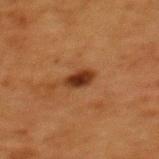Clinical impression:
No biopsy was performed on this lesion — it was imaged during a full skin examination and was not determined to be concerning.
Acquisition and patient details:
A female subject aged 48–52. The lesion's longest dimension is about 3 mm. The lesion is located on the mid back. Automated image analysis of the tile measured a mean CIELAB color near L≈29 a*≈21 b*≈30, a lesion–skin lightness drop of about 12, and a lesion-to-skin contrast of about 11.5 (normalized; higher = more distinct). And it measured a border-irregularity index near 2/10 and peripheral color asymmetry of about 0.5. This is a cross-polarized tile. A close-up tile cropped from a whole-body skin photograph, about 15 mm across.